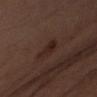follow-up = no biopsy performed (imaged during a skin exam)
TBP lesion metrics = an average lesion color of about L≈22 a*≈17 b*≈20 (CIELAB), roughly 6 lightness units darker than nearby skin, and a normalized lesion–skin contrast near 7.5; a border-irregularity rating of about 5/10, a within-lesion color-variation index near 1/10, and peripheral color asymmetry of about 0; a nevus-likeness score of about 50/100 and a lesion-detection confidence of about 100/100
lesion size = ~3.5 mm (longest diameter)
image source = total-body-photography crop, ~15 mm field of view
tile lighting = cross-polarized
site = the left arm
subject = male, in their 50s